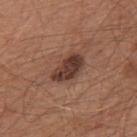| field | value |
|---|---|
| TBP lesion metrics | a classifier nevus-likeness of about 60/100 and a detector confidence of about 100 out of 100 that the crop contains a lesion |
| diameter | ≈4.5 mm |
| location | the mid back |
| acquisition | total-body-photography crop, ~15 mm field of view |
| subject | male, aged 63 to 67 |
| tile lighting | white-light |